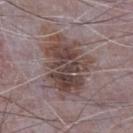  biopsy_status: not biopsied; imaged during a skin examination
  site: chest
  lighting: white-light
  automated_metrics:
    area_mm2_approx: 29.0
    eccentricity: 0.7
    shape_asymmetry: 0.25
    cielab_L: 43
    cielab_a: 15
    cielab_b: 18
    vs_skin_darker_L: 11.0
    color_variation_0_10: 6.5
    peripheral_color_asymmetry: 2.5
    nevus_likeness_0_100: 35
    lesion_detection_confidence_0_100: 95
  lesion_size:
    long_diameter_mm_approx: 7.5
  image:
    source: total-body photography crop
    field_of_view_mm: 15
  patient:
    sex: male
    age_approx: 65